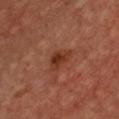Q: Was this lesion biopsied?
A: no biopsy performed (imaged during a skin exam)
Q: What is the anatomic site?
A: the chest
Q: What is the imaging modality?
A: total-body-photography crop, ~15 mm field of view
Q: Who is the patient?
A: female, aged around 45